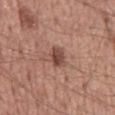Q: Was a biopsy performed?
A: imaged on a skin check; not biopsied
Q: Lesion size?
A: ≈3 mm
Q: What are the patient's age and sex?
A: male, aged around 55
Q: Illumination type?
A: white-light illumination
Q: Where on the body is the lesion?
A: the back
Q: How was this image acquired?
A: total-body-photography crop, ~15 mm field of view